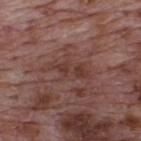Q: Is there a histopathology result?
A: imaged on a skin check; not biopsied
Q: Where on the body is the lesion?
A: the upper back
Q: Who is the patient?
A: male, aged approximately 70
Q: What kind of image is this?
A: total-body-photography crop, ~15 mm field of view
Q: Automated lesion metrics?
A: a mean CIELAB color near L≈38 a*≈20 b*≈23 and about 6 CIELAB-L* units darker than the surrounding skin; a border-irregularity index near 6.5/10 and a peripheral color-asymmetry measure near 1